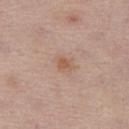Impression: Captured during whole-body skin photography for melanoma surveillance; the lesion was not biopsied. Image and clinical context: The lesion is located on the left thigh. The total-body-photography lesion software estimated a lesion color around L≈57 a*≈18 b*≈29 in CIELAB, roughly 7 lightness units darker than nearby skin, and a normalized border contrast of about 6. The analysis additionally found a classifier nevus-likeness of about 5/100 and a lesion-detection confidence of about 100/100. A female patient, aged 48 to 52. Imaged with white-light lighting. A roughly 15 mm field-of-view crop from a total-body skin photograph. About 2.5 mm across.